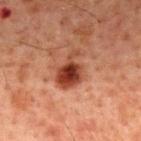Case summary:
• body site · the back
• tile lighting · cross-polarized
• imaging modality · ~15 mm tile from a whole-body skin photo
• subject · male, approximately 60 years of age